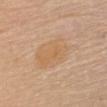| key | value |
|---|---|
| workup | no biopsy performed (imaged during a skin exam) |
| imaging modality | total-body-photography crop, ~15 mm field of view |
| lesion diameter | about 6.5 mm |
| subject | female, in their mid- to late 60s |
| TBP lesion metrics | a mean CIELAB color near L≈63 a*≈17 b*≈36; a border-irregularity index near 3/10 and peripheral color asymmetry of about 1; a nevus-likeness score of about 5/100 and lesion-presence confidence of about 100/100 |
| anatomic site | the front of the torso |
| lighting | white-light illumination |